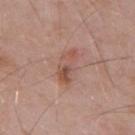Captured during whole-body skin photography for melanoma surveillance; the lesion was not biopsied. A roughly 15 mm field-of-view crop from a total-body skin photograph. This is a white-light tile. On the mid back. The lesion's longest dimension is about 4 mm. A male subject in their mid-50s.